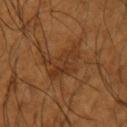Impression: Recorded during total-body skin imaging; not selected for excision or biopsy.